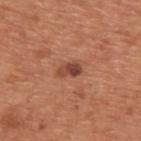No biopsy was performed on this lesion — it was imaged during a full skin examination and was not determined to be concerning. A region of skin cropped from a whole-body photographic capture, roughly 15 mm wide. About 3 mm across. From the upper back. A male subject, about 65 years old.